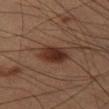| key | value |
|---|---|
| notes | no biopsy performed (imaged during a skin exam) |
| patient | male, aged 53–57 |
| lesion diameter | ≈3.5 mm |
| imaging modality | total-body-photography crop, ~15 mm field of view |
| lighting | cross-polarized illumination |
| body site | the right thigh |